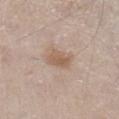Clinical impression:
Recorded during total-body skin imaging; not selected for excision or biopsy.